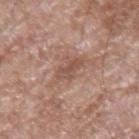Notes:
- patient · male, aged 58–62
- image source · ~15 mm tile from a whole-body skin photo
- automated lesion analysis · an area of roughly 5 mm², an outline eccentricity of about 0.85 (0 = round, 1 = elongated), and two-axis asymmetry of about 0.35; border irregularity of about 4 on a 0–10 scale, internal color variation of about 2.5 on a 0–10 scale, and radial color variation of about 1; a nevus-likeness score of about 0/100 and a detector confidence of about 95 out of 100 that the crop contains a lesion
- body site · the left forearm